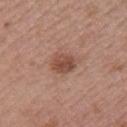{"biopsy_status": "not biopsied; imaged during a skin examination", "automated_metrics": {"eccentricity": 0.6, "cielab_L": 48, "cielab_a": 22, "cielab_b": 28, "vs_skin_darker_L": 10.0, "vs_skin_contrast_norm": 8.0, "nevus_likeness_0_100": 75, "lesion_detection_confidence_0_100": 100}, "image": {"source": "total-body photography crop", "field_of_view_mm": 15}, "site": "right upper arm", "patient": {"sex": "female", "age_approx": 50}, "lighting": "white-light", "lesion_size": {"long_diameter_mm_approx": 3.0}}